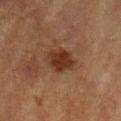Clinical impression: Imaged during a routine full-body skin examination; the lesion was not biopsied and no histopathology is available. Context: The lesion is located on the front of the torso. A male patient roughly 85 years of age or older. Captured under cross-polarized illumination. A 15 mm close-up tile from a total-body photography series done for melanoma screening. The total-body-photography lesion software estimated a footprint of about 8 mm², an outline eccentricity of about 0.5 (0 = round, 1 = elongated), and a symmetry-axis asymmetry near 0.15. The recorded lesion diameter is about 3.5 mm.